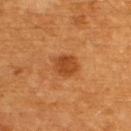workup=no biopsy performed (imaged during a skin exam) | site=the upper back | size=about 3 mm | patient=male, aged around 60 | automated metrics=an automated nevus-likeness rating near 95 out of 100 and lesion-presence confidence of about 100/100 | lighting=cross-polarized | image source=15 mm crop, total-body photography.